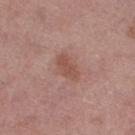Clinical impression:
The lesion was photographed on a routine skin check and not biopsied; there is no pathology result.
Context:
The lesion is on the right thigh. A female subject, aged 38 to 42. The total-body-photography lesion software estimated border irregularity of about 3 on a 0–10 scale. And it measured a nevus-likeness score of about 10/100. A roughly 15 mm field-of-view crop from a total-body skin photograph.